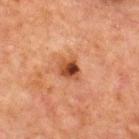biopsy status: imaged on a skin check; not biopsied
illumination: cross-polarized illumination
lesion diameter: about 2.5 mm
subject: male, aged 63–67
automated lesion analysis: a lesion area of about 5 mm², a shape eccentricity near 0.5, and a shape-asymmetry score of about 0.25 (0 = symmetric); a lesion color around L≈38 a*≈24 b*≈31 in CIELAB, about 12 CIELAB-L* units darker than the surrounding skin, and a lesion-to-skin contrast of about 10 (normalized; higher = more distinct); border irregularity of about 2 on a 0–10 scale, a color-variation rating of about 8/10, and radial color variation of about 3
anatomic site: the chest
acquisition: ~15 mm tile from a whole-body skin photo